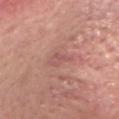Impression:
Recorded during total-body skin imaging; not selected for excision or biopsy.